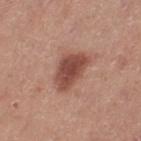Impression: Part of a total-body skin-imaging series; this lesion was reviewed on a skin check and was not flagged for biopsy. Acquisition and patient details: A 15 mm crop from a total-body photograph taken for skin-cancer surveillance. The tile uses white-light illumination. Measured at roughly 5 mm in maximum diameter. From the left thigh. A female subject aged 38–42.